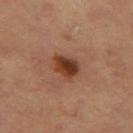  biopsy_status: not biopsied; imaged during a skin examination
  lighting: cross-polarized
  site: right leg
  patient:
    sex: female
    age_approx: 60
  lesion_size:
    long_diameter_mm_approx: 3.5
  image:
    source: total-body photography crop
    field_of_view_mm: 15
  automated_metrics:
    area_mm2_approx: 7.0
    eccentricity: 0.65
    shape_asymmetry: 0.2
    cielab_L: 39
    cielab_a: 25
    cielab_b: 31
    vs_skin_darker_L: 13.0
    vs_skin_contrast_norm: 10.5
    border_irregularity_0_10: 1.5
    nevus_likeness_0_100: 100